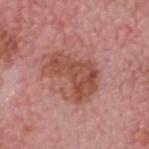workup: imaged on a skin check; not biopsied | size: ~6.5 mm (longest diameter) | body site: the head or neck | patient: male, in their mid-70s | illumination: white-light illumination | image source: 15 mm crop, total-body photography.